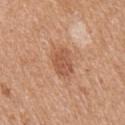Clinical impression:
This lesion was catalogued during total-body skin photography and was not selected for biopsy.
Context:
A roughly 15 mm field-of-view crop from a total-body skin photograph. Located on the right upper arm. Captured under white-light illumination. A female subject aged approximately 50.